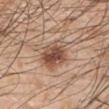No biopsy was performed on this lesion — it was imaged during a full skin examination and was not determined to be concerning.
A male subject aged 48–52.
This image is a 15 mm lesion crop taken from a total-body photograph.
From the left upper arm.